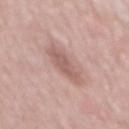Notes:
- workup: catalogued during a skin exam; not biopsied
- tile lighting: white-light
- subject: male, about 50 years old
- image source: ~15 mm crop, total-body skin-cancer survey
- location: the mid back
- automated lesion analysis: a mean CIELAB color near L≈59 a*≈20 b*≈23, roughly 10 lightness units darker than nearby skin, and a normalized lesion–skin contrast near 6.5; a nevus-likeness score of about 0/100 and a lesion-detection confidence of about 100/100
- size: ~6 mm (longest diameter)